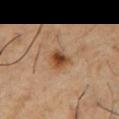workup — no biopsy performed (imaged during a skin exam)
subject — male, approximately 55 years of age
illumination — cross-polarized
site — the chest
automated lesion analysis — a mean CIELAB color near L≈45 a*≈21 b*≈34, about 12 CIELAB-L* units darker than the surrounding skin, and a normalized lesion–skin contrast near 9.5; a border-irregularity rating of about 2.5/10, a within-lesion color-variation index near 6/10, and a peripheral color-asymmetry measure near 2
diameter — ~2.5 mm (longest diameter)
image — ~15 mm crop, total-body skin-cancer survey